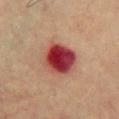This lesion was catalogued during total-body skin photography and was not selected for biopsy. From the chest. A roughly 15 mm field-of-view crop from a total-body skin photograph. A male subject aged 73 to 77. Imaged with cross-polarized lighting.